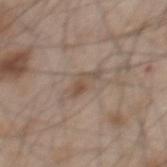follow-up: total-body-photography surveillance lesion; no biopsy | site: the back | illumination: white-light illumination | imaging modality: 15 mm crop, total-body photography | subject: male, about 45 years old.